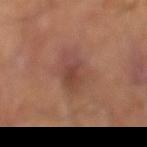Q: Was a biopsy performed?
A: total-body-photography surveillance lesion; no biopsy
Q: Lesion size?
A: ~4 mm (longest diameter)
Q: What lighting was used for the tile?
A: cross-polarized
Q: How was this image acquired?
A: ~15 mm crop, total-body skin-cancer survey
Q: Patient demographics?
A: male, aged 63–67
Q: Where on the body is the lesion?
A: the left lower leg